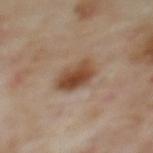Assessment: Captured during whole-body skin photography for melanoma surveillance; the lesion was not biopsied. Acquisition and patient details: A female patient aged 58 to 62. A close-up tile cropped from a whole-body skin photograph, about 15 mm across. Approximately 4 mm at its widest. The tile uses cross-polarized illumination. The lesion is on the upper back.